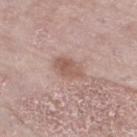biopsy status: imaged on a skin check; not biopsied | automated metrics: a border-irregularity index near 2/10, internal color variation of about 3 on a 0–10 scale, and peripheral color asymmetry of about 1; a detector confidence of about 100 out of 100 that the crop contains a lesion | acquisition: ~15 mm crop, total-body skin-cancer survey | lesion diameter: ≈4 mm | tile lighting: white-light illumination | location: the left lower leg | subject: female, aged 68 to 72.